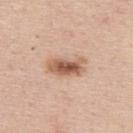Captured during whole-body skin photography for melanoma surveillance; the lesion was not biopsied. Automated image analysis of the tile measured a mean CIELAB color near L≈58 a*≈21 b*≈31. The analysis additionally found border irregularity of about 3 on a 0–10 scale, a within-lesion color-variation index near 6/10, and a peripheral color-asymmetry measure near 2. Imaged with white-light lighting. A male subject in their mid-40s. About 4.5 mm across. This image is a 15 mm lesion crop taken from a total-body photograph. The lesion is on the upper back.